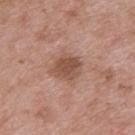Clinical summary:
About 3.5 mm across. The subject is a male approximately 50 years of age. This image is a 15 mm lesion crop taken from a total-body photograph. Automated tile analysis of the lesion measured an outline eccentricity of about 0.6 (0 = round, 1 = elongated) and a symmetry-axis asymmetry near 0.15. The analysis additionally found a border-irregularity rating of about 2/10 and peripheral color asymmetry of about 1. The software also gave an automated nevus-likeness rating near 10 out of 100 and a lesion-detection confidence of about 100/100. Captured under white-light illumination. On the upper back.